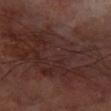Part of a total-body skin-imaging series; this lesion was reviewed on a skin check and was not flagged for biopsy.
A male patient aged 63 to 67.
The lesion-visualizer software estimated an area of roughly 100 mm², an outline eccentricity of about 0.85 (0 = round, 1 = elongated), and a symmetry-axis asymmetry near 0.3. It also reported border irregularity of about 7 on a 0–10 scale, a within-lesion color-variation index near 4.5/10, and a peripheral color-asymmetry measure near 1.5. It also reported a classifier nevus-likeness of about 0/100 and a lesion-detection confidence of about 80/100.
Measured at roughly 15.5 mm in maximum diameter.
Located on the left forearm.
A 15 mm close-up tile from a total-body photography series done for melanoma screening.
The tile uses cross-polarized illumination.